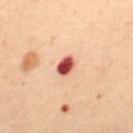{
  "image": {
    "source": "total-body photography crop",
    "field_of_view_mm": 15
  },
  "site": "mid back",
  "lighting": "cross-polarized",
  "patient": {
    "sex": "female",
    "age_approx": 50
  },
  "lesion_size": {
    "long_diameter_mm_approx": 2.5
  }
}